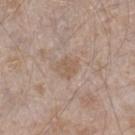Clinical impression:
Imaged during a routine full-body skin examination; the lesion was not biopsied and no histopathology is available.
Context:
On the leg. A region of skin cropped from a whole-body photographic capture, roughly 15 mm wide. The patient is a male aged around 45. Automated image analysis of the tile measured a lesion color around L≈57 a*≈15 b*≈28 in CIELAB, a lesion–skin lightness drop of about 6, and a normalized lesion–skin contrast near 5.5. The analysis additionally found a border-irregularity rating of about 2/10.